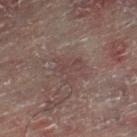Recorded during total-body skin imaging; not selected for excision or biopsy. This image is a 15 mm lesion crop taken from a total-body photograph. Captured under cross-polarized illumination. A male subject, approximately 60 years of age. The lesion is on the right lower leg. About 3.5 mm across.